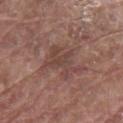{
  "biopsy_status": "not biopsied; imaged during a skin examination",
  "lesion_size": {
    "long_diameter_mm_approx": 4.5
  },
  "patient": {
    "sex": "male",
    "age_approx": 80
  },
  "lighting": "white-light",
  "site": "chest",
  "image": {
    "source": "total-body photography crop",
    "field_of_view_mm": 15
  }
}